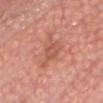The lesion was photographed on a routine skin check and not biopsied; there is no pathology result. A male subject aged 63 to 67. About 4.5 mm across. Located on the head or neck. This image is a 15 mm lesion crop taken from a total-body photograph. Automated image analysis of the tile measured border irregularity of about 6 on a 0–10 scale and internal color variation of about 2 on a 0–10 scale. And it measured an automated nevus-likeness rating near 0 out of 100 and a lesion-detection confidence of about 100/100. The tile uses white-light illumination.